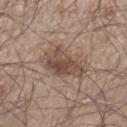- biopsy status: catalogued during a skin exam; not biopsied
- lesion diameter: about 4.5 mm
- image: 15 mm crop, total-body photography
- TBP lesion metrics: an area of roughly 13 mm²
- subject: male, approximately 45 years of age
- site: the right lower leg